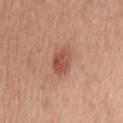workup = imaged on a skin check; not biopsied | image = total-body-photography crop, ~15 mm field of view | subject = male, aged around 45 | lesion size = ~3.5 mm (longest diameter) | body site = the head or neck | illumination = white-light.